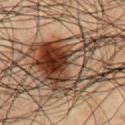| key | value |
|---|---|
| notes | catalogued during a skin exam; not biopsied |
| illumination | cross-polarized illumination |
| image | ~15 mm tile from a whole-body skin photo |
| site | the chest |
| lesion diameter | ~10.5 mm (longest diameter) |
| TBP lesion metrics | an area of roughly 36 mm², an outline eccentricity of about 0.7 (0 = round, 1 = elongated), and a shape-asymmetry score of about 0.55 (0 = symmetric) |
| patient | male, roughly 50 years of age |